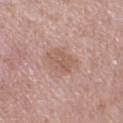notes: catalogued during a skin exam; not biopsied | lesion size: ~3.5 mm (longest diameter) | patient: female, aged 48–52 | acquisition: ~15 mm crop, total-body skin-cancer survey | illumination: white-light | location: the leg.